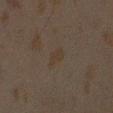Q: Is there a histopathology result?
A: no biopsy performed (imaged during a skin exam)
Q: What is the lesion's diameter?
A: ~2.5 mm (longest diameter)
Q: How was the tile lit?
A: cross-polarized illumination
Q: What is the imaging modality?
A: ~15 mm crop, total-body skin-cancer survey
Q: Lesion location?
A: the right upper arm
Q: Patient demographics?
A: male, aged 43–47